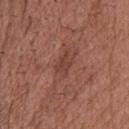The lesion was tiled from a total-body skin photograph and was not biopsied.
The lesion is located on the head or neck.
A male patient aged around 65.
Cropped from a total-body skin-imaging series; the visible field is about 15 mm.
Captured under white-light illumination.
The lesion-visualizer software estimated an area of roughly 4 mm², a shape eccentricity near 0.8, and two-axis asymmetry of about 0.4. And it measured a lesion color around L≈41 a*≈23 b*≈26 in CIELAB, a lesion–skin lightness drop of about 7, and a normalized border contrast of about 6. The software also gave an automated nevus-likeness rating near 5 out of 100 and a detector confidence of about 90 out of 100 that the crop contains a lesion.
The recorded lesion diameter is about 3 mm.